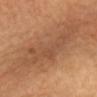{
  "biopsy_status": "not biopsied; imaged during a skin examination",
  "lighting": "cross-polarized",
  "automated_metrics": {
    "area_mm2_approx": 20.0,
    "eccentricity": 0.9,
    "shape_asymmetry": 0.5,
    "border_irregularity_0_10": 7.5,
    "color_variation_0_10": 2.5,
    "peripheral_color_asymmetry": 1.0
  },
  "site": "head or neck",
  "image": {
    "source": "total-body photography crop",
    "field_of_view_mm": 15
  },
  "lesion_size": {
    "long_diameter_mm_approx": 8.5
  },
  "patient": {
    "sex": "female",
    "age_approx": 65
  }
}